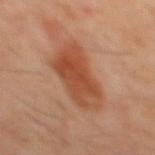The lesion was photographed on a routine skin check and not biopsied; there is no pathology result.